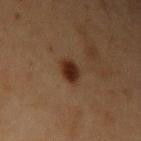biopsy_status: not biopsied; imaged during a skin examination
lighting: cross-polarized
site: left upper arm
image:
  source: total-body photography crop
  field_of_view_mm: 15
patient:
  sex: female
  age_approx: 40